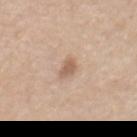- biopsy status — imaged on a skin check; not biopsied
- diameter — ≈2.5 mm
- tile lighting — white-light
- image source — 15 mm crop, total-body photography
- site — the front of the torso
- subject — male, aged around 65